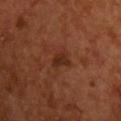  biopsy_status: not biopsied; imaged during a skin examination
  patient:
    sex: male
    age_approx: 55
  site: chest
  image:
    source: total-body photography crop
    field_of_view_mm: 15
  lesion_size:
    long_diameter_mm_approx: 2.5
  lighting: cross-polarized